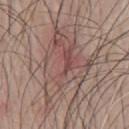Clinical impression:
This lesion was catalogued during total-body skin photography and was not selected for biopsy.
Clinical summary:
The lesion-visualizer software estimated a lesion color around L≈51 a*≈18 b*≈21 in CIELAB, roughly 8 lightness units darker than nearby skin, and a normalized border contrast of about 6. The software also gave an automated nevus-likeness rating near 0 out of 100. The lesion is on the chest. The subject is a male aged around 65. Longest diameter approximately 10.5 mm. A roughly 15 mm field-of-view crop from a total-body skin photograph.